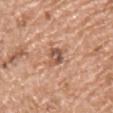The total-body-photography lesion software estimated a footprint of about 4 mm², an eccentricity of roughly 0.8, and a shape-asymmetry score of about 0.3 (0 = symmetric). And it measured roughly 12 lightness units darker than nearby skin. A 15 mm close-up tile from a total-body photography series done for melanoma screening. The lesion is on the chest. This is a white-light tile. Longest diameter approximately 3 mm. The patient is a male aged 53–57.